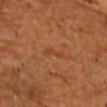The lesion was photographed on a routine skin check and not biopsied; there is no pathology result. A 15 mm close-up tile from a total-body photography series done for melanoma screening. The tile uses cross-polarized illumination. A male patient, aged around 60. The lesion is located on the front of the torso. Longest diameter approximately 2.5 mm.